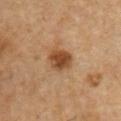Case summary:
- notes — no biopsy performed (imaged during a skin exam)
- patient — male, aged approximately 55
- automated lesion analysis — a lesion area of about 6.5 mm² and an outline eccentricity of about 0.55 (0 = round, 1 = elongated); border irregularity of about 1.5 on a 0–10 scale and a peripheral color-asymmetry measure near 1; an automated nevus-likeness rating near 95 out of 100 and lesion-presence confidence of about 100/100
- site — the front of the torso
- size — ~3 mm (longest diameter)
- acquisition — total-body-photography crop, ~15 mm field of view
- tile lighting — cross-polarized